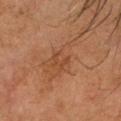workup=imaged on a skin check; not biopsied
lighting=cross-polarized
anatomic site=the head or neck
size=≈2.5 mm
patient=male, in their mid-60s
imaging modality=~15 mm tile from a whole-body skin photo